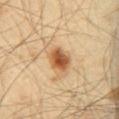follow-up = catalogued during a skin exam; not biopsied